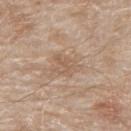The lesion was photographed on a routine skin check and not biopsied; there is no pathology result. From the upper back. Automated image analysis of the tile measured a lesion area of about 6.5 mm², an eccentricity of roughly 0.65, and two-axis asymmetry of about 0.4. The analysis additionally found a lesion color around L≈58 a*≈16 b*≈30 in CIELAB. The analysis additionally found a border-irregularity rating of about 4/10, a within-lesion color-variation index near 2.5/10, and radial color variation of about 1. A 15 mm crop from a total-body photograph taken for skin-cancer surveillance. A male patient, aged 78 to 82.